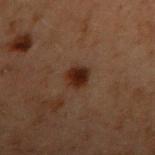Impression: The lesion was tiled from a total-body skin photograph and was not biopsied. Background: Captured under cross-polarized illumination. This image is a 15 mm lesion crop taken from a total-body photograph. On the right upper arm. The subject is a male about 60 years old.